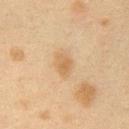{
  "biopsy_status": "not biopsied; imaged during a skin examination",
  "site": "right upper arm",
  "patient": {
    "sex": "female",
    "age_approx": 40
  },
  "lighting": "cross-polarized",
  "automated_metrics": {
    "eccentricity": 0.75,
    "shape_asymmetry": 0.25,
    "cielab_L": 52,
    "cielab_a": 15,
    "cielab_b": 33,
    "vs_skin_darker_L": 7.0,
    "vs_skin_contrast_norm": 6.0
  },
  "image": {
    "source": "total-body photography crop",
    "field_of_view_mm": 15
  }
}